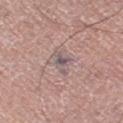Clinical impression:
No biopsy was performed on this lesion — it was imaged during a full skin examination and was not determined to be concerning.
Acquisition and patient details:
A male subject in their mid-70s. Automated tile analysis of the lesion measured border irregularity of about 5 on a 0–10 scale, a within-lesion color-variation index near 5/10, and radial color variation of about 1.5. It also reported an automated nevus-likeness rating near 0 out of 100. A close-up tile cropped from a whole-body skin photograph, about 15 mm across. On the left thigh. This is a white-light tile. The lesion's longest dimension is about 3.5 mm.